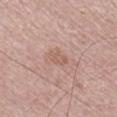follow-up = total-body-photography surveillance lesion; no biopsy | body site = the right lower leg | patient = male, aged 48–52 | imaging modality = 15 mm crop, total-body photography | tile lighting = white-light illumination.